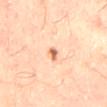Q: Was a biopsy performed?
A: catalogued during a skin exam; not biopsied
Q: What are the patient's age and sex?
A: male, roughly 55 years of age
Q: What is the anatomic site?
A: the mid back
Q: What kind of image is this?
A: 15 mm crop, total-body photography